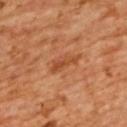No biopsy was performed on this lesion — it was imaged during a full skin examination and was not determined to be concerning. Measured at roughly 4 mm in maximum diameter. An algorithmic analysis of the crop reported a footprint of about 4.5 mm², an eccentricity of roughly 0.9, and a shape-asymmetry score of about 0.4 (0 = symmetric). The analysis additionally found a lesion–skin lightness drop of about 8 and a lesion-to-skin contrast of about 6.5 (normalized; higher = more distinct). The software also gave a border-irregularity index near 4.5/10 and a peripheral color-asymmetry measure near 1. A female patient, about 55 years old. The lesion is located on the upper back. Cropped from a whole-body photographic skin survey; the tile spans about 15 mm.